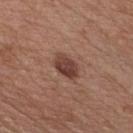Recorded during total-body skin imaging; not selected for excision or biopsy.
Automated image analysis of the tile measured a lesion area of about 6.5 mm², an eccentricity of roughly 0.8, and two-axis asymmetry of about 0.2. It also reported a border-irregularity rating of about 2/10 and radial color variation of about 1.5.
About 3.5 mm across.
A 15 mm close-up tile from a total-body photography series done for melanoma screening.
A male subject, roughly 55 years of age.
On the chest.
This is a white-light tile.